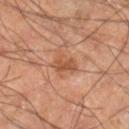Clinical impression:
This lesion was catalogued during total-body skin photography and was not selected for biopsy.
Context:
Automated image analysis of the tile measured a lesion area of about 5 mm² and a symmetry-axis asymmetry near 0.4. The software also gave a mean CIELAB color near L≈50 a*≈24 b*≈33, a lesion–skin lightness drop of about 9, and a normalized lesion–skin contrast near 7. The analysis additionally found a border-irregularity rating of about 3.5/10, internal color variation of about 2 on a 0–10 scale, and radial color variation of about 1. The lesion is located on the right lower leg. Measured at roughly 3 mm in maximum diameter. This is a cross-polarized tile. A male subject aged approximately 60. Cropped from a whole-body photographic skin survey; the tile spans about 15 mm.